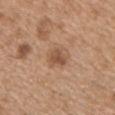Captured during whole-body skin photography for melanoma surveillance; the lesion was not biopsied. On the chest. This image is a 15 mm lesion crop taken from a total-body photograph. Approximately 3 mm at its widest. The patient is a male roughly 50 years of age.